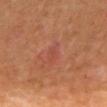size: ≈1.5 mm | automated metrics: peripheral color asymmetry of about 0; a lesion-detection confidence of about 95/100 | patient: male, in their 70s | anatomic site: the mid back | image: total-body-photography crop, ~15 mm field of view.